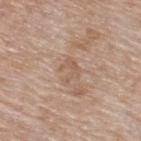| field | value |
|---|---|
| follow-up | catalogued during a skin exam; not biopsied |
| body site | the back |
| tile lighting | white-light illumination |
| subject | female, about 75 years old |
| acquisition | 15 mm crop, total-body photography |
| image-analysis metrics | an eccentricity of roughly 0.65; a mean CIELAB color near L≈57 a*≈17 b*≈29 and a normalized border contrast of about 5; a border-irregularity rating of about 7/10 and internal color variation of about 0 on a 0–10 scale |
| diameter | about 3 mm |